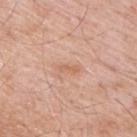  biopsy_status: not biopsied; imaged during a skin examination
  site: upper back
  lighting: white-light
  image:
    source: total-body photography crop
    field_of_view_mm: 15
  patient:
    sex: male
    age_approx: 65
  lesion_size:
    long_diameter_mm_approx: 2.5